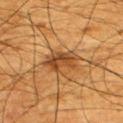Q: Was this lesion biopsied?
A: no biopsy performed (imaged during a skin exam)
Q: What is the lesion's diameter?
A: ~4 mm (longest diameter)
Q: Illumination type?
A: cross-polarized
Q: How was this image acquired?
A: 15 mm crop, total-body photography
Q: What are the patient's age and sex?
A: male, about 65 years old
Q: Lesion location?
A: the back
Q: What did automated image analysis measure?
A: a lesion area of about 9 mm² and an outline eccentricity of about 0.75 (0 = round, 1 = elongated); a mean CIELAB color near L≈39 a*≈20 b*≈34; a classifier nevus-likeness of about 65/100 and lesion-presence confidence of about 95/100